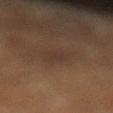biopsy status: imaged on a skin check; not biopsied | image: ~15 mm crop, total-body skin-cancer survey | patient: male, aged 58–62 | body site: the left lower leg | diameter: ≈4 mm | automated lesion analysis: a footprint of about 6.5 mm² and an eccentricity of roughly 0.85; a border-irregularity index near 3/10, internal color variation of about 1.5 on a 0–10 scale, and a peripheral color-asymmetry measure near 0.5 | illumination: cross-polarized.